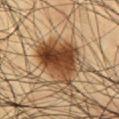Q: Is there a histopathology result?
A: total-body-photography surveillance lesion; no biopsy
Q: What are the patient's age and sex?
A: male, aged 58 to 62
Q: What did automated image analysis measure?
A: a lesion color around L≈43 a*≈20 b*≈34 in CIELAB, a lesion–skin lightness drop of about 18, and a lesion-to-skin contrast of about 13 (normalized; higher = more distinct); a border-irregularity index near 4/10, a color-variation rating of about 8/10, and radial color variation of about 3; an automated nevus-likeness rating near 100 out of 100 and lesion-presence confidence of about 100/100
Q: Illumination type?
A: cross-polarized
Q: What is the imaging modality?
A: ~15 mm tile from a whole-body skin photo
Q: Where on the body is the lesion?
A: the abdomen
Q: Lesion size?
A: ≈6 mm